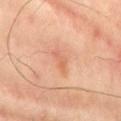workup: no biopsy performed (imaged during a skin exam); anatomic site: the lower back; illumination: cross-polarized; image source: total-body-photography crop, ~15 mm field of view; subject: male, aged around 65.